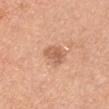Imaged during a routine full-body skin examination; the lesion was not biopsied and no histopathology is available. A 15 mm close-up tile from a total-body photography series done for melanoma screening. Longest diameter approximately 3 mm. Automated image analysis of the tile measured a lesion area of about 5.5 mm², a shape eccentricity near 0.55, and a shape-asymmetry score of about 0.25 (0 = symmetric). And it measured a lesion color around L≈61 a*≈23 b*≈34 in CIELAB, a lesion–skin lightness drop of about 10, and a lesion-to-skin contrast of about 6.5 (normalized; higher = more distinct). The analysis additionally found a border-irregularity rating of about 2.5/10, a color-variation rating of about 3/10, and peripheral color asymmetry of about 1. This is a white-light tile. Located on the right upper arm. A male patient aged 28–32.